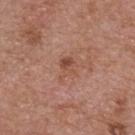| field | value |
|---|---|
| notes | no biopsy performed (imaged during a skin exam) |
| lesion size | about 3 mm |
| location | the chest |
| automated metrics | a shape eccentricity near 0.65 and a shape-asymmetry score of about 0.5 (0 = symmetric); a nevus-likeness score of about 0/100 and a lesion-detection confidence of about 100/100 |
| subject | male, in their mid-50s |
| image source | total-body-photography crop, ~15 mm field of view |
| illumination | white-light illumination |